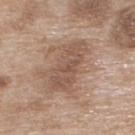imaging modality: ~15 mm crop, total-body skin-cancer survey | site: the upper back | lighting: white-light illumination | subject: female, in their mid- to late 70s | lesion diameter: about 6 mm.